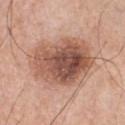Captured during whole-body skin photography for melanoma surveillance; the lesion was not biopsied.
The patient is a male about 50 years old.
A 15 mm close-up extracted from a 3D total-body photography capture.
The lesion is located on the chest.
The recorded lesion diameter is about 8 mm.
Automated image analysis of the tile measured an area of roughly 37 mm², an outline eccentricity of about 0.65 (0 = round, 1 = elongated), and two-axis asymmetry of about 0.15. And it measured an average lesion color of about L≈54 a*≈21 b*≈28 (CIELAB), roughly 14 lightness units darker than nearby skin, and a lesion-to-skin contrast of about 9.5 (normalized; higher = more distinct). The analysis additionally found a border-irregularity index near 2/10, internal color variation of about 8.5 on a 0–10 scale, and radial color variation of about 3.